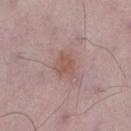From the left lower leg.
A male subject aged 48–52.
A 15 mm crop from a total-body photograph taken for skin-cancer surveillance.